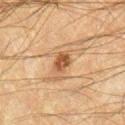notes: catalogued during a skin exam; not biopsied | size: about 2.5 mm | anatomic site: the right lower leg | patient: male, aged approximately 50 | image source: ~15 mm crop, total-body skin-cancer survey.